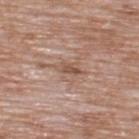  biopsy_status: not biopsied; imaged during a skin examination
  patient:
    sex: male
    age_approx: 60
  automated_metrics:
    border_irregularity_0_10: 3.5
    color_variation_0_10: 1.0
    peripheral_color_asymmetry: 0.0
  image:
    source: total-body photography crop
    field_of_view_mm: 15
  lesion_size:
    long_diameter_mm_approx: 3.0
  lighting: white-light
  site: upper back